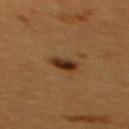{"biopsy_status": "not biopsied; imaged during a skin examination", "lighting": "cross-polarized", "patient": {"sex": "female", "age_approx": 55}, "site": "upper back", "image": {"source": "total-body photography crop", "field_of_view_mm": 15}}